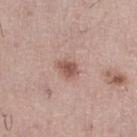{"lighting": "white-light", "patient": {"sex": "male", "age_approx": 55}, "image": {"source": "total-body photography crop", "field_of_view_mm": 15}, "lesion_size": {"long_diameter_mm_approx": 2.5}}